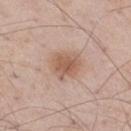Clinical impression:
The lesion was photographed on a routine skin check and not biopsied; there is no pathology result.
Context:
Approximately 3.5 mm at its widest. The patient is a male in their mid- to late 50s. The lesion is located on the leg. Cropped from a total-body skin-imaging series; the visible field is about 15 mm.